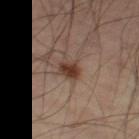The lesion was photographed on a routine skin check and not biopsied; there is no pathology result. A male patient, in their 50s. The lesion is on the abdomen. A roughly 15 mm field-of-view crop from a total-body skin photograph. The lesion's longest dimension is about 2.5 mm. This is a cross-polarized tile.